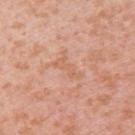Notes:
• notes: no biopsy performed (imaged during a skin exam)
• subject: female, aged approximately 40
• diameter: ~4 mm (longest diameter)
• image source: ~15 mm tile from a whole-body skin photo
• body site: the back
• lighting: white-light
• image-analysis metrics: a lesion–skin lightness drop of about 6 and a normalized lesion–skin contrast near 4.5; a within-lesion color-variation index near 0/10 and peripheral color asymmetry of about 0